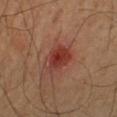<case>
<biopsy_status>not biopsied; imaged during a skin examination</biopsy_status>
<lighting>cross-polarized</lighting>
<lesion_size>
  <long_diameter_mm_approx>3.5</long_diameter_mm_approx>
</lesion_size>
<automated_metrics>
  <eccentricity>0.55</eccentricity>
  <border_irregularity_0_10>2.5</border_irregularity_0_10>
  <color_variation_0_10>5.0</color_variation_0_10>
  <peripheral_color_asymmetry>1.5</peripheral_color_asymmetry>
</automated_metrics>
<patient>
  <sex>male</sex>
  <age_approx>55</age_approx>
</patient>
<image>
  <source>total-body photography crop</source>
  <field_of_view_mm>15</field_of_view_mm>
</image>
<site>left lower leg</site>
</case>